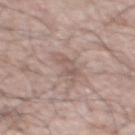Imaged during a routine full-body skin examination; the lesion was not biopsied and no histopathology is available.
The patient is a male aged approximately 65.
Captured under white-light illumination.
A 15 mm close-up extracted from a 3D total-body photography capture.
The lesion's longest dimension is about 4.5 mm.
The lesion is located on the chest.